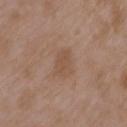Imaged during a routine full-body skin examination; the lesion was not biopsied and no histopathology is available. This is a white-light tile. The lesion is located on the left upper arm. A roughly 15 mm field-of-view crop from a total-body skin photograph. Longest diameter approximately 3 mm. A female patient, about 35 years old.